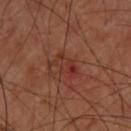biopsy status: catalogued during a skin exam; not biopsied | patient: male, aged around 60 | image: ~15 mm tile from a whole-body skin photo | size: about 3.5 mm | location: the upper back | automated metrics: a lesion area of about 6 mm² and a shape eccentricity near 0.85; a mean CIELAB color near L≈32 a*≈25 b*≈26; a border-irregularity rating of about 5.5/10 and internal color variation of about 8 on a 0–10 scale; an automated nevus-likeness rating near 0 out of 100 and a lesion-detection confidence of about 100/100.